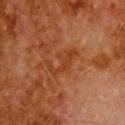No biopsy was performed on this lesion — it was imaged during a full skin examination and was not determined to be concerning.
The patient is a male aged around 80.
A 15 mm close-up extracted from a 3D total-body photography capture.
This is a cross-polarized tile.
The recorded lesion diameter is about 4.5 mm.
The lesion is located on the upper back.
Automated tile analysis of the lesion measured a mean CIELAB color near L≈31 a*≈22 b*≈31 and a lesion-to-skin contrast of about 5.5 (normalized; higher = more distinct). And it measured a border-irregularity rating of about 8/10, internal color variation of about 1.5 on a 0–10 scale, and peripheral color asymmetry of about 0.5. And it measured a nevus-likeness score of about 0/100 and a detector confidence of about 100 out of 100 that the crop contains a lesion.